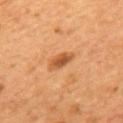  biopsy_status: not biopsied; imaged during a skin examination
  lighting: cross-polarized
  automated_metrics:
    cielab_L: 50
    cielab_a: 25
    cielab_b: 39
    vs_skin_contrast_norm: 8.0
  patient:
    sex: male
    age_approx: 55
  lesion_size:
    long_diameter_mm_approx: 3.5
  image:
    source: total-body photography crop
    field_of_view_mm: 15
  site: mid back